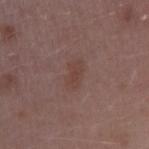No biopsy was performed on this lesion — it was imaged during a full skin examination and was not determined to be concerning. A roughly 15 mm field-of-view crop from a total-body skin photograph. The lesion is on the right upper arm. Approximately 3.5 mm at its widest. The subject is a female aged 43 to 47. Imaged with white-light lighting.